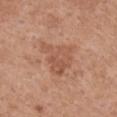<lesion>
  <biopsy_status>not biopsied; imaged during a skin examination</biopsy_status>
  <patient>
    <sex>female</sex>
    <age_approx>65</age_approx>
  </patient>
  <image>
    <source>total-body photography crop</source>
    <field_of_view_mm>15</field_of_view_mm>
  </image>
  <site>front of the torso</site>
</lesion>